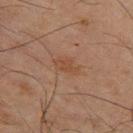  biopsy_status: not biopsied; imaged during a skin examination
  automated_metrics:
    eccentricity: 0.8
    shape_asymmetry: 0.35
    cielab_L: 37
    cielab_a: 17
    cielab_b: 26
    vs_skin_darker_L: 5.0
    vs_skin_contrast_norm: 5.5
    nevus_likeness_0_100: 0
    lesion_detection_confidence_0_100: 100
  patient:
    sex: male
    age_approx: 65
  image:
    source: total-body photography crop
    field_of_view_mm: 15
  lesion_size:
    long_diameter_mm_approx: 3.0
  site: leg
  lighting: cross-polarized